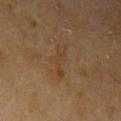Part of a total-body skin-imaging series; this lesion was reviewed on a skin check and was not flagged for biopsy. A close-up tile cropped from a whole-body skin photograph, about 15 mm across. From the left upper arm. Imaged with cross-polarized lighting. A female subject, aged approximately 80. Measured at roughly 4.5 mm in maximum diameter.